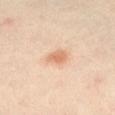Impression:
Part of a total-body skin-imaging series; this lesion was reviewed on a skin check and was not flagged for biopsy.
Image and clinical context:
This is a cross-polarized tile. A 15 mm close-up tile from a total-body photography series done for melanoma screening. A female patient aged 38 to 42. About 3 mm across. On the left thigh.